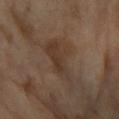Impression: Part of a total-body skin-imaging series; this lesion was reviewed on a skin check and was not flagged for biopsy. Image and clinical context: Imaged with cross-polarized lighting. A female patient about 70 years old. On the left forearm. A 15 mm close-up extracted from a 3D total-body photography capture.